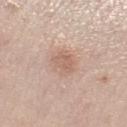Findings:
* notes · no biopsy performed (imaged during a skin exam)
* anatomic site · the left lower leg
* size · about 3.5 mm
* subject · female, aged 38–42
* imaging modality · ~15 mm crop, total-body skin-cancer survey
* lighting · white-light
* automated lesion analysis · a footprint of about 8.5 mm² and an outline eccentricity of about 0.5 (0 = round, 1 = elongated); a lesion color around L≈63 a*≈18 b*≈27 in CIELAB and roughly 8 lightness units darker than nearby skin